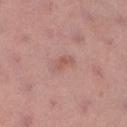Impression:
Imaged during a routine full-body skin examination; the lesion was not biopsied and no histopathology is available.
Background:
Imaged with white-light lighting. A female patient aged approximately 55. The lesion is located on the right lower leg. A lesion tile, about 15 mm wide, cut from a 3D total-body photograph. The lesion's longest dimension is about 3 mm.